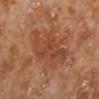follow-up=total-body-photography surveillance lesion; no biopsy | subject=male, in their 70s | automated lesion analysis=a lesion area of about 20 mm² and two-axis asymmetry of about 0.35; a mean CIELAB color near L≈43 a*≈24 b*≈31 and a lesion–skin lightness drop of about 7; a border-irregularity rating of about 5/10, internal color variation of about 4 on a 0–10 scale, and radial color variation of about 1.5; a classifier nevus-likeness of about 5/100 and a lesion-detection confidence of about 100/100 | anatomic site=the left lower leg | lesion size=≈7 mm | illumination=cross-polarized illumination | image=~15 mm crop, total-body skin-cancer survey.